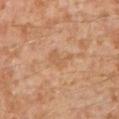biopsy_status: not biopsied; imaged during a skin examination
automated_metrics:
  area_mm2_approx: 4.0
  eccentricity: 0.85
  shape_asymmetry: 0.45
  nevus_likeness_0_100: 0
  lesion_detection_confidence_0_100: 100
image:
  source: total-body photography crop
  field_of_view_mm: 15
lighting: cross-polarized
site: left lower leg
patient:
  sex: male
  age_approx: 30